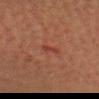This lesion was catalogued during total-body skin photography and was not selected for biopsy.
A roughly 15 mm field-of-view crop from a total-body skin photograph.
A male subject, aged 38 to 42.
The lesion is on the head or neck.